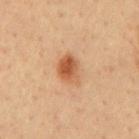Impression:
Captured during whole-body skin photography for melanoma surveillance; the lesion was not biopsied.
Background:
Located on the mid back. A region of skin cropped from a whole-body photographic capture, roughly 15 mm wide. The total-body-photography lesion software estimated an automated nevus-likeness rating near 100 out of 100 and lesion-presence confidence of about 100/100. A male subject, in their mid-60s. Captured under cross-polarized illumination.